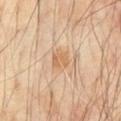{
  "lighting": "cross-polarized",
  "automated_metrics": {
    "area_mm2_approx": 5.0,
    "eccentricity": 0.5,
    "shape_asymmetry": 0.2,
    "cielab_L": 63,
    "cielab_a": 19,
    "cielab_b": 36,
    "vs_skin_darker_L": 7.0,
    "vs_skin_contrast_norm": 6.0,
    "nevus_likeness_0_100": 5,
    "lesion_detection_confidence_0_100": 100
  },
  "patient": {
    "sex": "male",
    "age_approx": 70
  },
  "image": {
    "source": "total-body photography crop",
    "field_of_view_mm": 15
  },
  "lesion_size": {
    "long_diameter_mm_approx": 2.5
  },
  "site": "front of the torso"
}